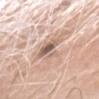- notes: no biopsy performed (imaged during a skin exam)
- illumination: white-light illumination
- patient: male, in their 80s
- imaging modality: ~15 mm tile from a whole-body skin photo
- location: the head or neck